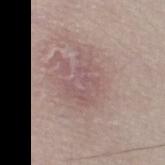biopsy status: imaged on a skin check; not biopsied | automated lesion analysis: a footprint of about 12 mm², an outline eccentricity of about 0.75 (0 = round, 1 = elongated), and a shape-asymmetry score of about 0.45 (0 = symmetric); border irregularity of about 6 on a 0–10 scale, a within-lesion color-variation index near 2.5/10, and peripheral color asymmetry of about 1; a classifier nevus-likeness of about 0/100 | lesion size: about 5 mm | imaging modality: 15 mm crop, total-body photography | patient: female, in their 60s | body site: the right thigh.